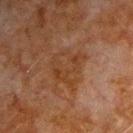follow-up=imaged on a skin check; not biopsied
automated lesion analysis=a lesion area of about 12 mm² and a shape eccentricity near 0.4; lesion-presence confidence of about 100/100
patient=male, about 80 years old
image source=~15 mm crop, total-body skin-cancer survey
size=~4 mm (longest diameter)
anatomic site=the chest